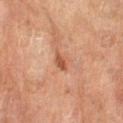| key | value |
|---|---|
| follow-up | catalogued during a skin exam; not biopsied |
| image source | ~15 mm tile from a whole-body skin photo |
| illumination | cross-polarized |
| patient | female, aged approximately 70 |
| lesion size | about 2.5 mm |
| body site | the right thigh |